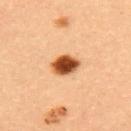This lesion was catalogued during total-body skin photography and was not selected for biopsy. The total-body-photography lesion software estimated a border-irregularity index near 1.5/10, internal color variation of about 6.5 on a 0–10 scale, and a peripheral color-asymmetry measure near 2. A 15 mm close-up extracted from a 3D total-body photography capture. About 3.5 mm across. From the upper back. A female patient, aged 33 to 37.